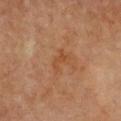Case summary:
* biopsy status — no biopsy performed (imaged during a skin exam)
* lighting — cross-polarized illumination
* acquisition — ~15 mm tile from a whole-body skin photo
* anatomic site — the chest
* patient — female, aged around 70
* image-analysis metrics — an outline eccentricity of about 0.8 (0 = round, 1 = elongated) and two-axis asymmetry of about 0.4; an average lesion color of about L≈42 a*≈21 b*≈32 (CIELAB), about 5 CIELAB-L* units darker than the surrounding skin, and a normalized lesion–skin contrast near 5